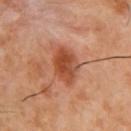Part of a total-body skin-imaging series; this lesion was reviewed on a skin check and was not flagged for biopsy. A lesion tile, about 15 mm wide, cut from a 3D total-body photograph. On the chest. A male patient, approximately 55 years of age. The lesion's longest dimension is about 4.5 mm. The lesion-visualizer software estimated a lesion-to-skin contrast of about 9 (normalized; higher = more distinct). It also reported border irregularity of about 1.5 on a 0–10 scale and peripheral color asymmetry of about 1.5. Captured under cross-polarized illumination.